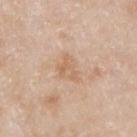The lesion was tiled from a total-body skin photograph and was not biopsied. The tile uses white-light illumination. The patient is a male aged around 75. The total-body-photography lesion software estimated a footprint of about 6 mm². And it measured a mean CIELAB color near L≈64 a*≈18 b*≈33 and about 7 CIELAB-L* units darker than the surrounding skin. Cropped from a total-body skin-imaging series; the visible field is about 15 mm. Located on the right upper arm. Longest diameter approximately 3.5 mm.